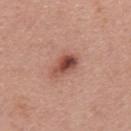The lesion-visualizer software estimated a classifier nevus-likeness of about 85/100 and a lesion-detection confidence of about 100/100. Located on the mid back. Imaged with white-light lighting. The subject is a male roughly 60 years of age. About 3.5 mm across. Cropped from a total-body skin-imaging series; the visible field is about 15 mm.